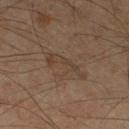Impression: The lesion was tiled from a total-body skin photograph and was not biopsied. Acquisition and patient details: The tile uses cross-polarized illumination. On the left thigh. The subject is a male about 60 years old. A 15 mm crop from a total-body photograph taken for skin-cancer surveillance. The total-body-photography lesion software estimated a footprint of about 6.5 mm², a shape eccentricity near 0.95, and two-axis asymmetry of about 0.5. And it measured a border-irregularity rating of about 7/10, a color-variation rating of about 2.5/10, and a peripheral color-asymmetry measure near 0.5.